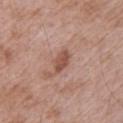Recorded during total-body skin imaging; not selected for excision or biopsy. An algorithmic analysis of the crop reported a footprint of about 4.5 mm² and two-axis asymmetry of about 0.2. The software also gave a mean CIELAB color near L≈51 a*≈23 b*≈27, about 11 CIELAB-L* units darker than the surrounding skin, and a lesion-to-skin contrast of about 7.5 (normalized; higher = more distinct). And it measured a classifier nevus-likeness of about 35/100 and a detector confidence of about 100 out of 100 that the crop contains a lesion. A male subject aged 63 to 67. On the left upper arm. Imaged with white-light lighting. Longest diameter approximately 3.5 mm. This image is a 15 mm lesion crop taken from a total-body photograph.